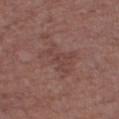Q: Is there a histopathology result?
A: imaged on a skin check; not biopsied
Q: Who is the patient?
A: male, in their mid-70s
Q: Where on the body is the lesion?
A: the right thigh
Q: What is the imaging modality?
A: 15 mm crop, total-body photography
Q: Automated lesion metrics?
A: an outline eccentricity of about 0.65 (0 = round, 1 = elongated) and a shape-asymmetry score of about 0.4 (0 = symmetric); a normalized border contrast of about 4.5; border irregularity of about 6 on a 0–10 scale, a within-lesion color-variation index near 2/10, and a peripheral color-asymmetry measure near 1
Q: How was the tile lit?
A: white-light